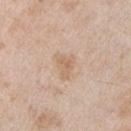This lesion was catalogued during total-body skin photography and was not selected for biopsy. A male subject roughly 55 years of age. Imaged with white-light lighting. A 15 mm crop from a total-body photograph taken for skin-cancer surveillance. From the arm.